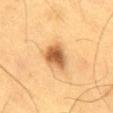biopsy status: total-body-photography surveillance lesion; no biopsy
imaging modality: 15 mm crop, total-body photography
TBP lesion metrics: an area of roughly 8.5 mm² and a shape-asymmetry score of about 0.25 (0 = symmetric); an automated nevus-likeness rating near 100 out of 100 and lesion-presence confidence of about 100/100
body site: the back
patient: male, in their 60s